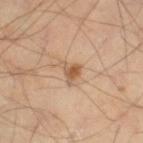{
  "biopsy_status": "not biopsied; imaged during a skin examination",
  "image": {
    "source": "total-body photography crop",
    "field_of_view_mm": 15
  },
  "patient": {
    "sex": "male",
    "age_approx": 45
  },
  "site": "left thigh"
}